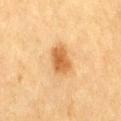Case summary:
- workup · imaged on a skin check; not biopsied
- TBP lesion metrics · an average lesion color of about L≈53 a*≈21 b*≈39 (CIELAB), roughly 11 lightness units darker than nearby skin, and a normalized border contrast of about 8.5; border irregularity of about 2 on a 0–10 scale and peripheral color asymmetry of about 1; a classifier nevus-likeness of about 100/100
- diameter · about 3.5 mm
- patient · female, roughly 55 years of age
- imaging modality · total-body-photography crop, ~15 mm field of view
- anatomic site · the right thigh